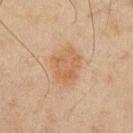workup: total-body-photography surveillance lesion; no biopsy
patient: male, in their mid-40s
imaging modality: ~15 mm crop, total-body skin-cancer survey
site: the chest
lighting: cross-polarized illumination